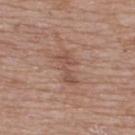notes: total-body-photography surveillance lesion; no biopsy | anatomic site: the back | lesion diameter: ≈4 mm | image-analysis metrics: an area of roughly 5.5 mm² | subject: male, aged 73 to 77 | tile lighting: white-light | imaging modality: ~15 mm crop, total-body skin-cancer survey.